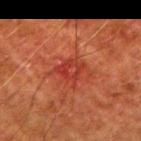Q: Is there a histopathology result?
A: total-body-photography surveillance lesion; no biopsy
Q: What did automated image analysis measure?
A: a footprint of about 6 mm² and two-axis asymmetry of about 0.3; an average lesion color of about L≈32 a*≈31 b*≈28 (CIELAB), roughly 6 lightness units darker than nearby skin, and a normalized border contrast of about 6; an automated nevus-likeness rating near 0 out of 100 and a detector confidence of about 95 out of 100 that the crop contains a lesion
Q: What kind of image is this?
A: ~15 mm tile from a whole-body skin photo
Q: What is the anatomic site?
A: the right upper arm
Q: What is the lesion's diameter?
A: ≈3 mm
Q: What are the patient's age and sex?
A: male, approximately 80 years of age
Q: Illumination type?
A: cross-polarized